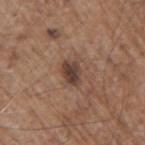<lesion>
  <biopsy_status>not biopsied; imaged during a skin examination</biopsy_status>
  <site>right upper arm</site>
  <lesion_size>
    <long_diameter_mm_approx>3.0</long_diameter_mm_approx>
  </lesion_size>
  <patient>
    <sex>male</sex>
    <age_approx>70</age_approx>
  </patient>
  <automated_metrics>
    <area_mm2_approx>5.5</area_mm2_approx>
    <eccentricity>0.7</eccentricity>
    <shape_asymmetry>0.3</shape_asymmetry>
    <cielab_L>41</cielab_L>
    <cielab_a>17</cielab_a>
    <cielab_b>24</cielab_b>
    <vs_skin_darker_L>11.0</vs_skin_darker_L>
    <vs_skin_contrast_norm>9.5</vs_skin_contrast_norm>
    <border_irregularity_0_10>3.0</border_irregularity_0_10>
    <color_variation_0_10>3.5</color_variation_0_10>
    <peripheral_color_asymmetry>1.0</peripheral_color_asymmetry>
    <nevus_likeness_0_100>55</nevus_likeness_0_100>
    <lesion_detection_confidence_0_100>100</lesion_detection_confidence_0_100>
  </automated_metrics>
  <image>
    <source>total-body photography crop</source>
    <field_of_view_mm>15</field_of_view_mm>
  </image>
</lesion>